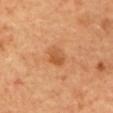patient = female, approximately 60 years of age | imaging modality = ~15 mm crop, total-body skin-cancer survey | site = the upper back.